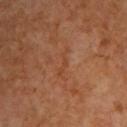<tbp_lesion>
<biopsy_status>not biopsied; imaged during a skin examination</biopsy_status>
<patient>
  <sex>male</sex>
  <age_approx>60</age_approx>
</patient>
<lesion_size>
  <long_diameter_mm_approx>3.5</long_diameter_mm_approx>
</lesion_size>
<site>chest</site>
<lighting>cross-polarized</lighting>
<image>
  <source>total-body photography crop</source>
  <field_of_view_mm>15</field_of_view_mm>
</image>
</tbp_lesion>